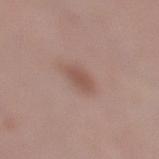Assessment:
The lesion was tiled from a total-body skin photograph and was not biopsied.
Image and clinical context:
The lesion is on the leg. This image is a 15 mm lesion crop taken from a total-body photograph. The lesion's longest dimension is about 3 mm. A female patient roughly 40 years of age.